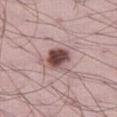Captured during whole-body skin photography for melanoma surveillance; the lesion was not biopsied.
A 15 mm close-up tile from a total-body photography series done for melanoma screening.
Captured under white-light illumination.
The total-body-photography lesion software estimated a footprint of about 7 mm², a shape eccentricity near 0.7, and two-axis asymmetry of about 0.2. It also reported an average lesion color of about L≈46 a*≈20 b*≈19 (CIELAB), roughly 18 lightness units darker than nearby skin, and a normalized lesion–skin contrast near 12.5. And it measured an automated nevus-likeness rating near 95 out of 100 and lesion-presence confidence of about 100/100.
The patient is a male aged around 35.
The lesion is located on the right thigh.